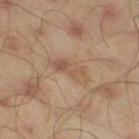Case summary:
- notes: catalogued during a skin exam; not biopsied
- automated metrics: a symmetry-axis asymmetry near 0.3; an average lesion color of about L≈53 a*≈17 b*≈29 (CIELAB), about 7 CIELAB-L* units darker than the surrounding skin, and a normalized border contrast of about 5
- tile lighting: cross-polarized illumination
- location: the right thigh
- acquisition: ~15 mm crop, total-body skin-cancer survey
- size: about 4.5 mm
- subject: male, roughly 45 years of age